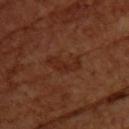biopsy_status: not biopsied; imaged during a skin examination
site: upper back
image:
  source: total-body photography crop
  field_of_view_mm: 15
patient:
  sex: female
  age_approx: 55
lesion_size:
  long_diameter_mm_approx: 4.0